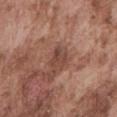Captured during whole-body skin photography for melanoma surveillance; the lesion was not biopsied.